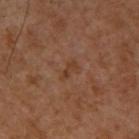No biopsy was performed on this lesion — it was imaged during a full skin examination and was not determined to be concerning.
A male subject aged 53 to 57.
A 15 mm close-up tile from a total-body photography series done for melanoma screening.
Imaged with cross-polarized lighting.
Located on the right upper arm.